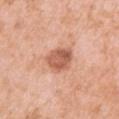Assessment: No biopsy was performed on this lesion — it was imaged during a full skin examination and was not determined to be concerning.